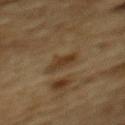Assessment:
The lesion was photographed on a routine skin check and not biopsied; there is no pathology result.
Context:
On the mid back. A male subject, aged approximately 85 or older. A 15 mm crop from a total-body photograph taken for skin-cancer surveillance. This is a cross-polarized tile.